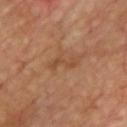This lesion was catalogued during total-body skin photography and was not selected for biopsy.